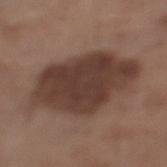Assessment:
Recorded during total-body skin imaging; not selected for excision or biopsy.
Image and clinical context:
From the left lower leg. A 15 mm close-up extracted from a 3D total-body photography capture. This is a white-light tile. A male subject in their mid- to late 60s. About 10 mm across.